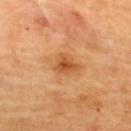A close-up tile cropped from a whole-body skin photograph, about 15 mm across.
From the upper back.
The lesion-visualizer software estimated an area of roughly 4.5 mm², a shape eccentricity near 0.6, and two-axis asymmetry of about 0.4. And it measured a within-lesion color-variation index near 3/10 and radial color variation of about 1.
Approximately 2.5 mm at its widest.
The patient is a female about 60 years old.